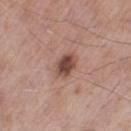Clinical impression:
The lesion was photographed on a routine skin check and not biopsied; there is no pathology result.
Acquisition and patient details:
A male subject, in their mid- to late 50s. On the left thigh. Cropped from a whole-body photographic skin survey; the tile spans about 15 mm. The recorded lesion diameter is about 3 mm.